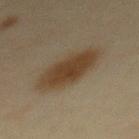| key | value |
|---|---|
| biopsy status | imaged on a skin check; not biopsied |
| imaging modality | ~15 mm tile from a whole-body skin photo |
| diameter | about 7 mm |
| subject | female, aged 43 to 47 |
| site | the back |
| lighting | cross-polarized illumination |
| automated lesion analysis | a footprint of about 20 mm² and a symmetry-axis asymmetry near 0.15; an automated nevus-likeness rating near 100 out of 100 |